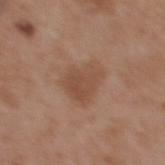anatomic site = the upper back
tile lighting = white-light illumination
acquisition = ~15 mm crop, total-body skin-cancer survey
patient = female, approximately 35 years of age
lesion size = ≈4.5 mm